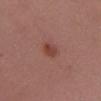Assessment:
The lesion was tiled from a total-body skin photograph and was not biopsied.
Clinical summary:
The patient is a male roughly 40 years of age. From the mid back. This is a white-light tile. The recorded lesion diameter is about 2.5 mm. A 15 mm close-up tile from a total-body photography series done for melanoma screening.